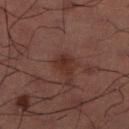Recorded during total-body skin imaging; not selected for excision or biopsy. Approximately 3 mm at its widest. A male patient, aged approximately 60. A 15 mm close-up extracted from a 3D total-body photography capture.